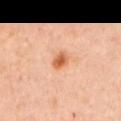The lesion was tiled from a total-body skin photograph and was not biopsied. Approximately 2.5 mm at its widest. The total-body-photography lesion software estimated a footprint of about 4 mm², a shape eccentricity near 0.6, and a symmetry-axis asymmetry near 0.2. The analysis additionally found a border-irregularity rating of about 1.5/10 and peripheral color asymmetry of about 1.5. And it measured an automated nevus-likeness rating near 95 out of 100 and lesion-presence confidence of about 100/100. The lesion is located on the left upper arm. A male subject, aged around 45. This image is a 15 mm lesion crop taken from a total-body photograph. This is a cross-polarized tile.